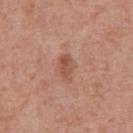biopsy status=no biopsy performed (imaged during a skin exam) | image source=~15 mm tile from a whole-body skin photo | location=the chest | subject=male, aged approximately 70.